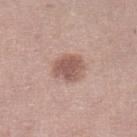follow-up — no biopsy performed (imaged during a skin exam) | site — the left lower leg | size — ≈3.5 mm | tile lighting — white-light | automated lesion analysis — a footprint of about 8.5 mm² and an outline eccentricity of about 0.55 (0 = round, 1 = elongated); a lesion color around L≈55 a*≈20 b*≈24 in CIELAB and about 12 CIELAB-L* units darker than the surrounding skin; a border-irregularity index near 2/10 and radial color variation of about 1; a classifier nevus-likeness of about 65/100 and a lesion-detection confidence of about 100/100 | image source — 15 mm crop, total-body photography | subject — female, aged 48–52.